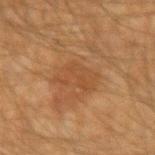| feature | finding |
|---|---|
| notes | no biopsy performed (imaged during a skin exam) |
| subject | male, about 50 years old |
| acquisition | ~15 mm crop, total-body skin-cancer survey |
| location | the right forearm |
| lesion size | about 4.5 mm |
| tile lighting | cross-polarized |